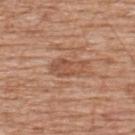The lesion was tiled from a total-body skin photograph and was not biopsied. From the upper back. Cropped from a whole-body photographic skin survey; the tile spans about 15 mm. A male subject, aged around 60. Imaged with white-light lighting. The total-body-photography lesion software estimated a lesion–skin lightness drop of about 9. The analysis additionally found a border-irregularity rating of about 3/10, internal color variation of about 3.5 on a 0–10 scale, and peripheral color asymmetry of about 1.5. It also reported a nevus-likeness score of about 0/100 and a lesion-detection confidence of about 100/100.